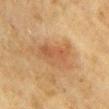follow-up: imaged on a skin check; not biopsied | automated metrics: a shape eccentricity near 0.9 and two-axis asymmetry of about 0.3; an average lesion color of about L≈49 a*≈20 b*≈34 (CIELAB) and a lesion–skin lightness drop of about 7; a within-lesion color-variation index near 3.5/10 and radial color variation of about 1; a classifier nevus-likeness of about 0/100 | subject: female, approximately 55 years of age | location: the right upper arm | acquisition: ~15 mm tile from a whole-body skin photo | lighting: cross-polarized | lesion diameter: ~5.5 mm (longest diameter).